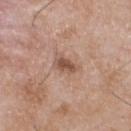Q: Was a biopsy performed?
A: imaged on a skin check; not biopsied
Q: How large is the lesion?
A: about 3 mm
Q: What is the anatomic site?
A: the chest
Q: Who is the patient?
A: male, about 50 years old
Q: What kind of image is this?
A: total-body-photography crop, ~15 mm field of view
Q: What lighting was used for the tile?
A: white-light